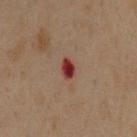Q: Is there a histopathology result?
A: total-body-photography surveillance lesion; no biopsy
Q: Lesion location?
A: the chest
Q: What is the imaging modality?
A: ~15 mm tile from a whole-body skin photo
Q: What is the lesion's diameter?
A: ~2.5 mm (longest diameter)
Q: How was the tile lit?
A: cross-polarized
Q: Automated lesion metrics?
A: a footprint of about 3.5 mm², an outline eccentricity of about 0.8 (0 = round, 1 = elongated), and a symmetry-axis asymmetry near 0.2; a lesion color around L≈33 a*≈29 b*≈24 in CIELAB, a lesion–skin lightness drop of about 14, and a normalized border contrast of about 12.5; an automated nevus-likeness rating near 0 out of 100 and a lesion-detection confidence of about 100/100
Q: What are the patient's age and sex?
A: male, approximately 60 years of age